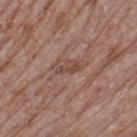This lesion was catalogued during total-body skin photography and was not selected for biopsy.
The lesion-visualizer software estimated a border-irregularity rating of about 4.5/10, a within-lesion color-variation index near 0.5/10, and peripheral color asymmetry of about 0.
Captured under white-light illumination.
A male patient, aged approximately 80.
The lesion's longest dimension is about 3 mm.
Located on the left thigh.
A 15 mm close-up tile from a total-body photography series done for melanoma screening.